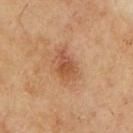Q: Is there a histopathology result?
A: no biopsy performed (imaged during a skin exam)
Q: What are the patient's age and sex?
A: male, aged 68 to 72
Q: How was this image acquired?
A: ~15 mm tile from a whole-body skin photo
Q: What is the anatomic site?
A: the right upper arm
Q: Lesion size?
A: ≈3.5 mm
Q: Illumination type?
A: cross-polarized illumination